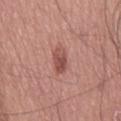Clinical impression:
No biopsy was performed on this lesion — it was imaged during a full skin examination and was not determined to be concerning.
Clinical summary:
The lesion is on the lower back. A 15 mm close-up extracted from a 3D total-body photography capture. Automated tile analysis of the lesion measured a within-lesion color-variation index near 4/10. The software also gave a classifier nevus-likeness of about 80/100 and a detector confidence of about 100 out of 100 that the crop contains a lesion. A male subject aged approximately 70. Longest diameter approximately 3.5 mm.